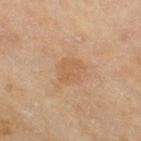The lesion is on the right thigh. A 15 mm close-up extracted from a 3D total-body photography capture. The subject is a female aged around 60. The lesion-visualizer software estimated an area of roughly 6 mm², a shape eccentricity near 0.45, and two-axis asymmetry of about 0.3. The analysis additionally found a mean CIELAB color near L≈53 a*≈18 b*≈33, a lesion–skin lightness drop of about 5, and a lesion-to-skin contrast of about 4.5 (normalized; higher = more distinct). The analysis additionally found a border-irregularity index near 3/10, a color-variation rating of about 2/10, and radial color variation of about 0.5. Imaged with cross-polarized lighting.